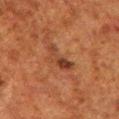Clinical impression:
Captured during whole-body skin photography for melanoma surveillance; the lesion was not biopsied.
Image and clinical context:
About 4 mm across. Located on the left lower leg. This is a cross-polarized tile. A 15 mm crop from a total-body photograph taken for skin-cancer surveillance. A male patient approximately 80 years of age.